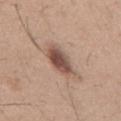{
  "biopsy_status": "not biopsied; imaged during a skin examination",
  "site": "mid back",
  "lighting": "white-light",
  "patient": {
    "sex": "male",
    "age_approx": 40
  },
  "image": {
    "source": "total-body photography crop",
    "field_of_view_mm": 15
  }
}